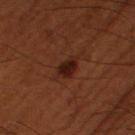imaging modality: ~15 mm tile from a whole-body skin photo
body site: the right thigh
subject: male, aged 48–52